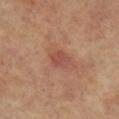Impression:
This lesion was catalogued during total-body skin photography and was not selected for biopsy.
Acquisition and patient details:
Captured under cross-polarized illumination. A 15 mm close-up tile from a total-body photography series done for melanoma screening. The lesion is on the left leg. The patient is a female aged 63 to 67.